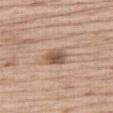* notes — total-body-photography surveillance lesion; no biopsy
* image source — ~15 mm crop, total-body skin-cancer survey
* lesion diameter — ≈3 mm
* subject — male, approximately 70 years of age
* automated metrics — an area of roughly 5.5 mm², a shape eccentricity near 0.7, and a symmetry-axis asymmetry near 0.25; a lesion-detection confidence of about 100/100
* anatomic site — the right thigh